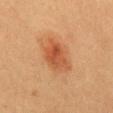size: ≈5 mm
lighting: cross-polarized illumination
location: the back
image source: ~15 mm tile from a whole-body skin photo
patient: female, roughly 20 years of age